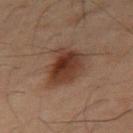Case summary:
- biopsy status: no biopsy performed (imaged during a skin exam)
- body site: the abdomen
- subject: male, aged 48 to 52
- illumination: cross-polarized
- lesion size: about 5.5 mm
- image: ~15 mm tile from a whole-body skin photo
- image-analysis metrics: an eccentricity of roughly 0.65 and two-axis asymmetry of about 0.25; a classifier nevus-likeness of about 95/100 and a lesion-detection confidence of about 100/100